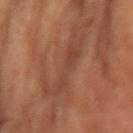No biopsy was performed on this lesion — it was imaged during a full skin examination and was not determined to be concerning. Automated tile analysis of the lesion measured an area of roughly 12 mm². A 15 mm close-up extracted from a 3D total-body photography capture. This is a cross-polarized tile. About 6 mm across. The lesion is on the left forearm. The subject is a female aged around 80.